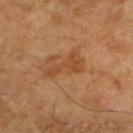Recorded during total-body skin imaging; not selected for excision or biopsy.
The patient is a male roughly 80 years of age.
A 15 mm crop from a total-body photograph taken for skin-cancer surveillance.
From the right thigh.
The recorded lesion diameter is about 4.5 mm.